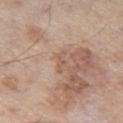| feature | finding |
|---|---|
| follow-up | catalogued during a skin exam; not biopsied |
| body site | the left thigh |
| subject | male, aged 58 to 62 |
| lesion size | about 2.5 mm |
| automated metrics | a nevus-likeness score of about 0/100 and a lesion-detection confidence of about 90/100 |
| tile lighting | white-light illumination |
| image | 15 mm crop, total-body photography |